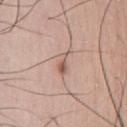| field | value |
|---|---|
| site | the front of the torso |
| tile lighting | white-light illumination |
| image source | 15 mm crop, total-body photography |
| patient | male, aged 73 to 77 |
| diameter | ~3 mm (longest diameter) |